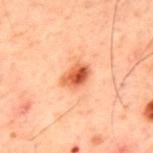A male subject, aged approximately 60. A 15 mm close-up tile from a total-body photography series done for melanoma screening. The lesion is on the upper back.